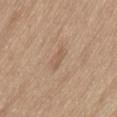<record>
<biopsy_status>not biopsied; imaged during a skin examination</biopsy_status>
<patient>
  <sex>male</sex>
  <age_approx>70</age_approx>
</patient>
<lighting>white-light</lighting>
<lesion_size>
  <long_diameter_mm_approx>2.5</long_diameter_mm_approx>
</lesion_size>
<site>back</site>
<image>
  <source>total-body photography crop</source>
  <field_of_view_mm>15</field_of_view_mm>
</image>
</record>